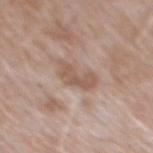automated metrics: an area of roughly 7 mm², an outline eccentricity of about 0.8 (0 = round, 1 = elongated), and a symmetry-axis asymmetry near 0.4; a lesion color around L≈56 a*≈17 b*≈27 in CIELAB, about 9 CIELAB-L* units darker than the surrounding skin, and a normalized lesion–skin contrast near 6.5; radial color variation of about 0.5; a classifier nevus-likeness of about 0/100
location: the back
illumination: white-light illumination
patient: male, aged 48–52
image source: ~15 mm crop, total-body skin-cancer survey